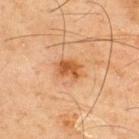follow-up = catalogued during a skin exam; not biopsied | lesion size = about 3.5 mm | body site = the back | imaging modality = ~15 mm tile from a whole-body skin photo | subject = male, aged around 45 | illumination = cross-polarized.